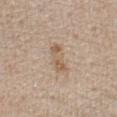{
  "biopsy_status": "not biopsied; imaged during a skin examination",
  "image": {
    "source": "total-body photography crop",
    "field_of_view_mm": 15
  },
  "patient": {
    "sex": "male",
    "age_approx": 75
  },
  "lighting": "white-light",
  "automated_metrics": {
    "eccentricity": 0.95,
    "shape_asymmetry": 0.25
  },
  "site": "chest"
}